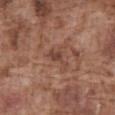Notes:
• workup: catalogued during a skin exam; not biopsied
• tile lighting: white-light illumination
• TBP lesion metrics: an area of roughly 3.5 mm², an outline eccentricity of about 0.9 (0 = round, 1 = elongated), and a symmetry-axis asymmetry near 0.45
• subject: male, approximately 75 years of age
• site: the abdomen
• image source: ~15 mm tile from a whole-body skin photo